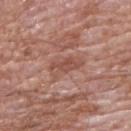Imaged during a routine full-body skin examination; the lesion was not biopsied and no histopathology is available. A 15 mm crop from a total-body photograph taken for skin-cancer surveillance. On the upper back. Automated image analysis of the tile measured roughly 8 lightness units darker than nearby skin and a normalized lesion–skin contrast near 6. It also reported a border-irregularity rating of about 3.5/10 and radial color variation of about 1. A male patient, aged 58 to 62. The recorded lesion diameter is about 4 mm. Imaged with white-light lighting.